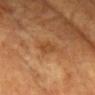follow-up = imaged on a skin check; not biopsied, subject = in their 60s, image = ~15 mm tile from a whole-body skin photo, location = the head or neck.